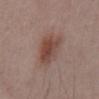Clinical impression:
Recorded during total-body skin imaging; not selected for excision or biopsy.
Clinical summary:
The recorded lesion diameter is about 4.5 mm. A male patient aged 53–57. The lesion is on the front of the torso. Imaged with white-light lighting. A close-up tile cropped from a whole-body skin photograph, about 15 mm across.